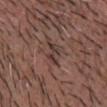No biopsy was performed on this lesion — it was imaged during a full skin examination and was not determined to be concerning.
The tile uses white-light illumination.
Approximately 3 mm at its widest.
On the chest.
The subject is a male aged 63–67.
Cropped from a total-body skin-imaging series; the visible field is about 15 mm.